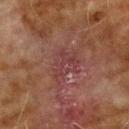{"biopsy_status": "not biopsied; imaged during a skin examination", "patient": {"sex": "male", "age_approx": 70}, "lesion_size": {"long_diameter_mm_approx": 5.0}, "lighting": "cross-polarized", "site": "right upper arm", "image": {"source": "total-body photography crop", "field_of_view_mm": 15}}